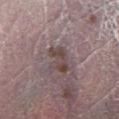Part of a total-body skin-imaging series; this lesion was reviewed on a skin check and was not flagged for biopsy.
Cropped from a whole-body photographic skin survey; the tile spans about 15 mm.
Located on the left leg.
A female subject aged 78 to 82.